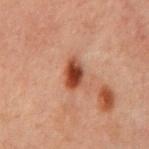notes: imaged on a skin check; not biopsied | image: 15 mm crop, total-body photography | patient: male, approximately 60 years of age | diameter: ≈3.5 mm | automated lesion analysis: a mean CIELAB color near L≈35 a*≈22 b*≈27, roughly 13 lightness units darker than nearby skin, and a normalized lesion–skin contrast near 11; an automated nevus-likeness rating near 100 out of 100 and a detector confidence of about 100 out of 100 that the crop contains a lesion | location: the chest.